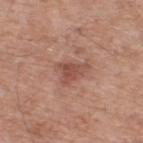<case>
  <site>upper back</site>
  <lesion_size>
    <long_diameter_mm_approx>3.5</long_diameter_mm_approx>
  </lesion_size>
  <lighting>white-light</lighting>
  <image>
    <source>total-body photography crop</source>
    <field_of_view_mm>15</field_of_view_mm>
  </image>
  <patient>
    <sex>male</sex>
    <age_approx>55</age_approx>
  </patient>
  <automated_metrics>
    <area_mm2_approx>5.5</area_mm2_approx>
    <eccentricity>0.75</eccentricity>
    <shape_asymmetry>0.5</shape_asymmetry>
    <lesion_detection_confidence_0_100>100</lesion_detection_confidence_0_100>
  </automated_metrics>
</case>